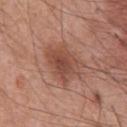notes: total-body-photography surveillance lesion; no biopsy | location: the chest | subject: male, aged approximately 55 | acquisition: total-body-photography crop, ~15 mm field of view.